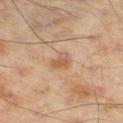| key | value |
|---|---|
| patient | male, in their mid- to late 50s |
| imaging modality | 15 mm crop, total-body photography |
| body site | the leg |